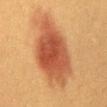workup: catalogued during a skin exam; not biopsied
site: the chest
image: total-body-photography crop, ~15 mm field of view
patient: female, aged 38–42